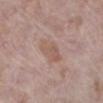Clinical impression:
No biopsy was performed on this lesion — it was imaged during a full skin examination and was not determined to be concerning.
Context:
Automated image analysis of the tile measured a border-irregularity index near 3/10, a color-variation rating of about 2/10, and radial color variation of about 0.5. A lesion tile, about 15 mm wide, cut from a 3D total-body photograph. Measured at roughly 3.5 mm in maximum diameter. Captured under white-light illumination. From the right lower leg. A female subject, approximately 70 years of age.